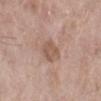- automated lesion analysis · an area of roughly 6 mm², an outline eccentricity of about 0.55 (0 = round, 1 = elongated), and a symmetry-axis asymmetry near 0.3; a border-irregularity rating of about 2.5/10 and a color-variation rating of about 2/10
- size · ~3 mm (longest diameter)
- patient · female, aged 73–77
- anatomic site · the left lower leg
- image · ~15 mm tile from a whole-body skin photo
- lighting · white-light illumination